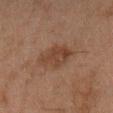| feature | finding |
|---|---|
| follow-up | total-body-photography surveillance lesion; no biopsy |
| illumination | cross-polarized |
| patient | male, aged 43–47 |
| acquisition | 15 mm crop, total-body photography |
| lesion diameter | about 5 mm |
| site | the right forearm |